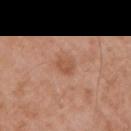This lesion was catalogued during total-body skin photography and was not selected for biopsy.
A male subject aged 53–57.
Located on the right upper arm.
A 15 mm crop from a total-body photograph taken for skin-cancer surveillance.
An algorithmic analysis of the crop reported a lesion area of about 4 mm² and two-axis asymmetry of about 0.25. And it measured about 8 CIELAB-L* units darker than the surrounding skin and a normalized lesion–skin contrast near 6. The analysis additionally found a nevus-likeness score of about 5/100.
The lesion's longest dimension is about 3 mm.